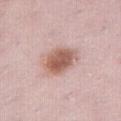biopsy status: catalogued during a skin exam; not biopsied
tile lighting: white-light
diameter: about 4.5 mm
site: the right thigh
acquisition: ~15 mm tile from a whole-body skin photo
patient: female, about 30 years old